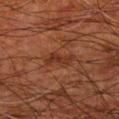Clinical impression: Recorded during total-body skin imaging; not selected for excision or biopsy. Image and clinical context: Captured under cross-polarized illumination. A close-up tile cropped from a whole-body skin photograph, about 15 mm across. Located on the left forearm. A male patient, in their 80s.